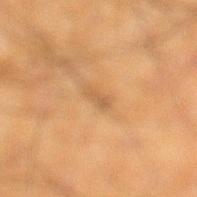Q: Is there a histopathology result?
A: total-body-photography surveillance lesion; no biopsy
Q: How was the tile lit?
A: cross-polarized illumination
Q: What is the anatomic site?
A: the left lower leg
Q: Lesion size?
A: ≈3 mm
Q: What did automated image analysis measure?
A: an eccentricity of roughly 0.95
Q: What kind of image is this?
A: total-body-photography crop, ~15 mm field of view
Q: What are the patient's age and sex?
A: male, in their 50s